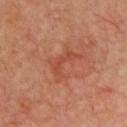<tbp_lesion>
<biopsy_status>not biopsied; imaged during a skin examination</biopsy_status>
<image>
  <source>total-body photography crop</source>
  <field_of_view_mm>15</field_of_view_mm>
</image>
<patient>
  <sex>male</sex>
  <age_approx>65</age_approx>
</patient>
<site>chest</site>
<lighting>cross-polarized</lighting>
<lesion_size>
  <long_diameter_mm_approx>4.5</long_diameter_mm_approx>
</lesion_size>
</tbp_lesion>